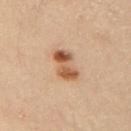Impression: Part of a total-body skin-imaging series; this lesion was reviewed on a skin check and was not flagged for biopsy. Context: Automated image analysis of the tile measured a classifier nevus-likeness of about 95/100 and a lesion-detection confidence of about 100/100. The lesion is located on the right upper arm. This image is a 15 mm lesion crop taken from a total-body photograph. This is a cross-polarized tile. A male subject, approximately 40 years of age. Measured at roughly 4.5 mm in maximum diameter.